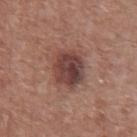• follow-up · total-body-photography surveillance lesion; no biopsy
• illumination · white-light illumination
• automated lesion analysis · lesion-presence confidence of about 100/100
• lesion size · ≈4 mm
• imaging modality · 15 mm crop, total-body photography
• patient · male, in their mid- to late 70s
• site · the abdomen